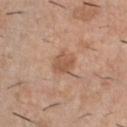| field | value |
|---|---|
| workup | imaged on a skin check; not biopsied |
| site | the chest |
| image-analysis metrics | a lesion area of about 5.5 mm², an eccentricity of roughly 0.5, and a shape-asymmetry score of about 0.2 (0 = symmetric); roughly 9 lightness units darker than nearby skin and a normalized border contrast of about 6.5 |
| image source | ~15 mm crop, total-body skin-cancer survey |
| patient | male, aged around 40 |
| diameter | ≈3 mm |